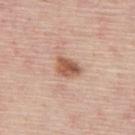No biopsy was performed on this lesion — it was imaged during a full skin examination and was not determined to be concerning. A lesion tile, about 15 mm wide, cut from a 3D total-body photograph. Imaged with white-light lighting. A male patient, in their mid- to late 40s. The lesion is located on the mid back. Longest diameter approximately 3 mm.